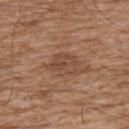Q: Was a biopsy performed?
A: catalogued during a skin exam; not biopsied
Q: What are the patient's age and sex?
A: male, roughly 60 years of age
Q: Where on the body is the lesion?
A: the chest
Q: What is the imaging modality?
A: total-body-photography crop, ~15 mm field of view
Q: What is the lesion's diameter?
A: ~4 mm (longest diameter)